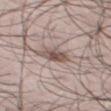{
  "biopsy_status": "not biopsied; imaged during a skin examination",
  "image": {
    "source": "total-body photography crop",
    "field_of_view_mm": 15
  },
  "site": "back",
  "lighting": "white-light",
  "patient": {
    "sex": "male",
    "age_approx": 45
  },
  "lesion_size": {
    "long_diameter_mm_approx": 2.5
  }
}